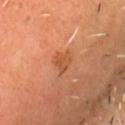No biopsy was performed on this lesion — it was imaged during a full skin examination and was not determined to be concerning. A male patient, aged around 55. From the head or neck. A region of skin cropped from a whole-body photographic capture, roughly 15 mm wide. Captured under cross-polarized illumination.